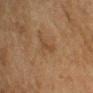Recorded during total-body skin imaging; not selected for excision or biopsy. A female subject about 55 years old. Automated tile analysis of the lesion measured an average lesion color of about L≈39 a*≈16 b*≈30 (CIELAB), a lesion–skin lightness drop of about 6, and a normalized lesion–skin contrast near 5.5. The analysis additionally found a border-irregularity rating of about 4.5/10, internal color variation of about 0 on a 0–10 scale, and radial color variation of about 0. From the left upper arm. Measured at roughly 3 mm in maximum diameter. A close-up tile cropped from a whole-body skin photograph, about 15 mm across. Captured under cross-polarized illumination.